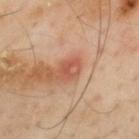Impression:
This lesion was catalogued during total-body skin photography and was not selected for biopsy.
Clinical summary:
Imaged with cross-polarized lighting. Automated tile analysis of the lesion measured an area of roughly 5 mm² and an eccentricity of roughly 0.75. The software also gave border irregularity of about 2 on a 0–10 scale, internal color variation of about 5.5 on a 0–10 scale, and peripheral color asymmetry of about 2. The patient is a male approximately 55 years of age. The lesion's longest dimension is about 3 mm. The lesion is on the mid back. This image is a 15 mm lesion crop taken from a total-body photograph.